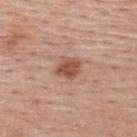Impression:
Part of a total-body skin-imaging series; this lesion was reviewed on a skin check and was not flagged for biopsy.
Context:
A 15 mm close-up tile from a total-body photography series done for melanoma screening. The patient is a female aged around 65. Automated image analysis of the tile measured an area of roughly 5.5 mm², an outline eccentricity of about 0.55 (0 = round, 1 = elongated), and a shape-asymmetry score of about 0.2 (0 = symmetric). The software also gave a classifier nevus-likeness of about 80/100 and a lesion-detection confidence of about 100/100. The lesion is on the back. Approximately 3 mm at its widest.